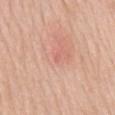Acquisition and patient details: Cropped from a total-body skin-imaging series; the visible field is about 15 mm. A female patient, roughly 70 years of age. On the mid back. The lesion's longest dimension is about 1 mm. The lesion-visualizer software estimated an average lesion color of about L≈63 a*≈27 b*≈29 (CIELAB), a lesion–skin lightness drop of about 5, and a normalized border contrast of about 3.5. It also reported a border-irregularity index near 4/10. Conclusion: Histopathological examination showed an atypical melanocytic neoplasm, classified as a lesion of indeterminate malignant potential.